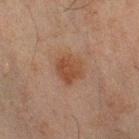This lesion was catalogued during total-body skin photography and was not selected for biopsy. A male patient, aged around 45. A roughly 15 mm field-of-view crop from a total-body skin photograph. Measured at roughly 3.5 mm in maximum diameter. From the right lower leg. This is a cross-polarized tile.